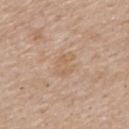Assessment: Captured during whole-body skin photography for melanoma surveillance; the lesion was not biopsied. Acquisition and patient details: A 15 mm crop from a total-body photograph taken for skin-cancer surveillance. This is a white-light tile. The lesion is located on the mid back. A male patient, about 55 years old.